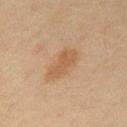Q: Lesion size?
A: ~4.5 mm (longest diameter)
Q: Lesion location?
A: the mid back
Q: Who is the patient?
A: male, aged 43 to 47
Q: What did automated image analysis measure?
A: a footprint of about 8 mm², a shape eccentricity near 0.9, and a shape-asymmetry score of about 0.25 (0 = symmetric)
Q: What lighting was used for the tile?
A: cross-polarized illumination
Q: What kind of image is this?
A: 15 mm crop, total-body photography